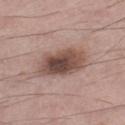  biopsy_status: not biopsied; imaged during a skin examination
  lesion_size:
    long_diameter_mm_approx: 5.5
  site: left lower leg
  automated_metrics:
    cielab_L: 48
    cielab_a: 18
    cielab_b: 23
    vs_skin_darker_L: 15.0
    vs_skin_contrast_norm: 10.5
    border_irregularity_0_10: 2.0
    color_variation_0_10: 6.0
  image:
    source: total-body photography crop
    field_of_view_mm: 15
  patient:
    sex: male
    age_approx: 50
  lighting: white-light